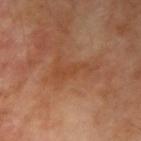The lesion was photographed on a routine skin check and not biopsied; there is no pathology result. Captured under cross-polarized illumination. The total-body-photography lesion software estimated an area of roughly 4 mm², an outline eccentricity of about 0.95 (0 = round, 1 = elongated), and a symmetry-axis asymmetry near 0.4. The software also gave an average lesion color of about L≈44 a*≈23 b*≈34 (CIELAB) and a lesion-to-skin contrast of about 5 (normalized; higher = more distinct). The software also gave a classifier nevus-likeness of about 0/100 and a detector confidence of about 85 out of 100 that the crop contains a lesion. From the right upper arm. A lesion tile, about 15 mm wide, cut from a 3D total-body photograph. The patient is a male roughly 70 years of age.